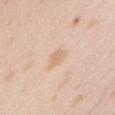Notes:
* biopsy status: catalogued during a skin exam; not biopsied
* image: ~15 mm crop, total-body skin-cancer survey
* body site: the head or neck
* tile lighting: white-light
* size: about 3 mm
* subject: female, aged 23–27